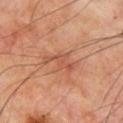Assessment: Part of a total-body skin-imaging series; this lesion was reviewed on a skin check and was not flagged for biopsy.